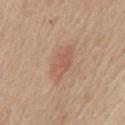illumination = white-light
subject = male, aged approximately 65
site = the chest
diameter = ~5 mm (longest diameter)
imaging modality = ~15 mm crop, total-body skin-cancer survey